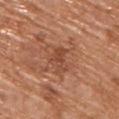  biopsy_status: not biopsied; imaged during a skin examination
  image:
    source: total-body photography crop
    field_of_view_mm: 15
  lesion_size:
    long_diameter_mm_approx: 3.0
  lighting: white-light
  site: upper back
  patient:
    sex: male
    age_approx: 70